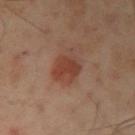Assessment:
This lesion was catalogued during total-body skin photography and was not selected for biopsy.
Clinical summary:
A male patient approximately 50 years of age. The recorded lesion diameter is about 4 mm. Automated tile analysis of the lesion measured a lesion area of about 9 mm², a shape eccentricity near 0.6, and a symmetry-axis asymmetry near 0.25. It also reported an average lesion color of about L≈41 a*≈23 b*≈28 (CIELAB) and a lesion-to-skin contrast of about 7 (normalized; higher = more distinct). The analysis additionally found a classifier nevus-likeness of about 100/100 and lesion-presence confidence of about 100/100. A 15 mm close-up extracted from a 3D total-body photography capture. The lesion is on the arm.